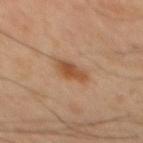follow-up = total-body-photography surveillance lesion; no biopsy
patient = male, in their mid-40s
image source = ~15 mm crop, total-body skin-cancer survey
illumination = cross-polarized
anatomic site = the mid back
lesion size = ~4 mm (longest diameter)